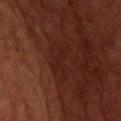<record>
<biopsy_status>not biopsied; imaged during a skin examination</biopsy_status>
<lesion_size>
  <long_diameter_mm_approx>4.5</long_diameter_mm_approx>
</lesion_size>
<patient>
  <sex>male</sex>
  <age_approx>60</age_approx>
</patient>
<site>chest</site>
<lighting>cross-polarized</lighting>
<image>
  <source>total-body photography crop</source>
  <field_of_view_mm>15</field_of_view_mm>
</image>
</record>